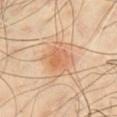Q: Was this lesion biopsied?
A: imaged on a skin check; not biopsied
Q: What is the lesion's diameter?
A: ~3 mm (longest diameter)
Q: What are the patient's age and sex?
A: male, roughly 65 years of age
Q: What kind of image is this?
A: ~15 mm crop, total-body skin-cancer survey
Q: What is the anatomic site?
A: the front of the torso
Q: What did automated image analysis measure?
A: an area of roughly 6 mm² and an eccentricity of roughly 0.55; an average lesion color of about L≈63 a*≈24 b*≈37 (CIELAB), about 7 CIELAB-L* units darker than the surrounding skin, and a normalized lesion–skin contrast near 5.5; a border-irregularity index near 2/10, a within-lesion color-variation index near 3/10, and a peripheral color-asymmetry measure near 1; a classifier nevus-likeness of about 100/100